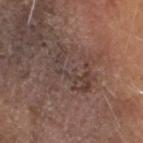Impression:
Imaged during a routine full-body skin examination; the lesion was not biopsied and no histopathology is available.
Context:
A male subject aged around 55. The lesion-visualizer software estimated a lesion area of about 9.5 mm² and a shape eccentricity near 0.9. It also reported a lesion color around L≈39 a*≈15 b*≈20 in CIELAB, a lesion–skin lightness drop of about 6, and a lesion-to-skin contrast of about 6 (normalized; higher = more distinct). The analysis additionally found a border-irregularity rating of about 9.5/10 and peripheral color asymmetry of about 1. It also reported a classifier nevus-likeness of about 0/100 and a lesion-detection confidence of about 75/100. This is a white-light tile. Cropped from a total-body skin-imaging series; the visible field is about 15 mm. Located on the head or neck.